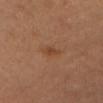Notes:
– workup · imaged on a skin check; not biopsied
– image · ~15 mm tile from a whole-body skin photo
– subject · female, in their mid-40s
– anatomic site · the head or neck